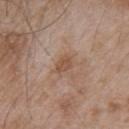workup: total-body-photography surveillance lesion; no biopsy | image: total-body-photography crop, ~15 mm field of view | lighting: white-light illumination | image-analysis metrics: a nevus-likeness score of about 0/100 and a lesion-detection confidence of about 100/100 | size: ≈2.5 mm | subject: male, aged around 55 | location: the upper back.